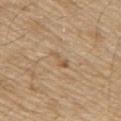biopsy status: total-body-photography surveillance lesion; no biopsy
automated lesion analysis: a lesion area of about 2.5 mm², a shape eccentricity near 0.9, and two-axis asymmetry of about 0.4; a lesion color around L≈56 a*≈16 b*≈35 in CIELAB, a lesion–skin lightness drop of about 8, and a normalized lesion–skin contrast near 6
diameter: ~2.5 mm (longest diameter)
image: 15 mm crop, total-body photography
body site: the arm
subject: male, aged 68–72
illumination: white-light illumination